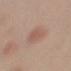| feature | finding |
|---|---|
| workup | no biopsy performed (imaged during a skin exam) |
| image | 15 mm crop, total-body photography |
| location | the left upper arm |
| illumination | white-light illumination |
| lesion diameter | ~2.5 mm (longest diameter) |
| patient | male, aged 48 to 52 |